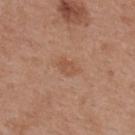Clinical impression:
Part of a total-body skin-imaging series; this lesion was reviewed on a skin check and was not flagged for biopsy.
Clinical summary:
The subject is a female aged approximately 40. The lesion is located on the upper back. This is a white-light tile. This image is a 15 mm lesion crop taken from a total-body photograph.